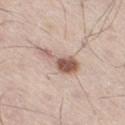Part of a total-body skin-imaging series; this lesion was reviewed on a skin check and was not flagged for biopsy.
Captured under white-light illumination.
Measured at roughly 5 mm in maximum diameter.
Located on the right thigh.
The total-body-photography lesion software estimated a footprint of about 8 mm², a shape eccentricity near 0.9, and a shape-asymmetry score of about 0.5 (0 = symmetric). And it measured a mean CIELAB color near L≈58 a*≈18 b*≈25, about 15 CIELAB-L* units darker than the surrounding skin, and a normalized lesion–skin contrast near 9.5. It also reported internal color variation of about 4 on a 0–10 scale and radial color variation of about 1.5.
The patient is a male aged approximately 55.
A 15 mm close-up tile from a total-body photography series done for melanoma screening.